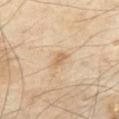Part of a total-body skin-imaging series; this lesion was reviewed on a skin check and was not flagged for biopsy. A male patient, aged 68 to 72. On the abdomen. The lesion's longest dimension is about 2.5 mm. A close-up tile cropped from a whole-body skin photograph, about 15 mm across. Automated image analysis of the tile measured a lesion area of about 3 mm² and a shape eccentricity near 0.8. And it measured a classifier nevus-likeness of about 5/100 and a lesion-detection confidence of about 100/100. The tile uses cross-polarized illumination.